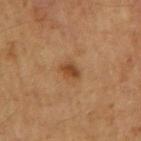This lesion was catalogued during total-body skin photography and was not selected for biopsy. A 15 mm crop from a total-body photograph taken for skin-cancer surveillance. On the right upper arm. A male patient, aged 58–62. The tile uses cross-polarized illumination.